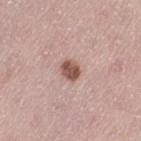Imaged during a routine full-body skin examination; the lesion was not biopsied and no histopathology is available.
The total-body-photography lesion software estimated a lesion color around L≈53 a*≈21 b*≈25 in CIELAB, a lesion–skin lightness drop of about 14, and a normalized lesion–skin contrast near 10.
A close-up tile cropped from a whole-body skin photograph, about 15 mm across.
Imaged with white-light lighting.
A female subject aged 38 to 42.
The lesion is on the leg.
Approximately 2.5 mm at its widest.